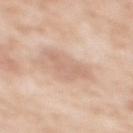Clinical impression:
Recorded during total-body skin imaging; not selected for excision or biopsy.
Image and clinical context:
Longest diameter approximately 4.5 mm. This is a white-light tile. Automated tile analysis of the lesion measured an area of roughly 8 mm² and a shape eccentricity near 0.8. The software also gave a nevus-likeness score of about 0/100. A 15 mm crop from a total-body photograph taken for skin-cancer surveillance. The lesion is located on the upper back. The subject is a female roughly 40 years of age.